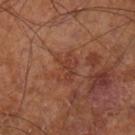Imaged during a routine full-body skin examination; the lesion was not biopsied and no histopathology is available. Cropped from a whole-body photographic skin survey; the tile spans about 15 mm. Imaged with cross-polarized lighting. From the leg. The subject is a male approximately 70 years of age. Longest diameter approximately 3.5 mm.